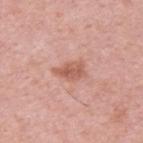Imaged during a routine full-body skin examination; the lesion was not biopsied and no histopathology is available. Automated image analysis of the tile measured an average lesion color of about L≈58 a*≈25 b*≈29 (CIELAB), roughly 10 lightness units darker than nearby skin, and a lesion-to-skin contrast of about 7 (normalized; higher = more distinct). The analysis additionally found a classifier nevus-likeness of about 0/100 and lesion-presence confidence of about 100/100. A male patient aged around 50. Measured at roughly 3.5 mm in maximum diameter. A close-up tile cropped from a whole-body skin photograph, about 15 mm across. Located on the upper back. Captured under white-light illumination.